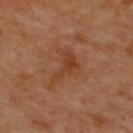notes: imaged on a skin check; not biopsied
image source: ~15 mm tile from a whole-body skin photo
TBP lesion metrics: an area of roughly 8 mm² and an outline eccentricity of about 0.8 (0 = round, 1 = elongated)
diameter: ≈4.5 mm
subject: roughly 65 years of age
site: the upper back